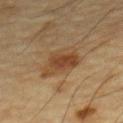Part of a total-body skin-imaging series; this lesion was reviewed on a skin check and was not flagged for biopsy.
About 4.5 mm across.
The lesion is on the chest.
A male subject about 85 years old or older.
This is a cross-polarized tile.
Cropped from a total-body skin-imaging series; the visible field is about 15 mm.